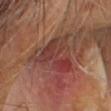biopsy status = imaged on a skin check; not biopsied
lighting = cross-polarized illumination
lesion size = ≈6 mm
imaging modality = ~15 mm crop, total-body skin-cancer survey
patient = male, aged 58–62
anatomic site = the head or neck